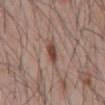| field | value |
|---|---|
| biopsy status | no biopsy performed (imaged during a skin exam) |
| patient | male, aged 43–47 |
| size | about 3 mm |
| tile lighting | white-light |
| TBP lesion metrics | an area of roughly 3.5 mm² and a shape eccentricity near 0.85; a mean CIELAB color near L≈45 a*≈19 b*≈25 |
| acquisition | ~15 mm tile from a whole-body skin photo |
| site | the mid back |